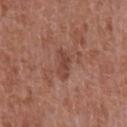No biopsy was performed on this lesion — it was imaged during a full skin examination and was not determined to be concerning. On the front of the torso. This image is a 15 mm lesion crop taken from a total-body photograph. A male subject aged 63 to 67.